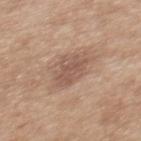Measured at roughly 4 mm in maximum diameter.
A female subject roughly 40 years of age.
Located on the mid back.
A close-up tile cropped from a whole-body skin photograph, about 15 mm across.
Captured under white-light illumination.